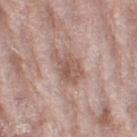The lesion was tiled from a total-body skin photograph and was not biopsied. The lesion is on the right thigh. A female subject, approximately 70 years of age. About 4.5 mm across. A 15 mm close-up extracted from a 3D total-body photography capture.